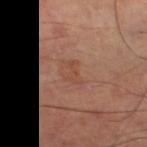| field | value |
|---|---|
| notes | no biopsy performed (imaged during a skin exam) |
| location | the left thigh |
| imaging modality | ~15 mm tile from a whole-body skin photo |
| patient | male, in their mid-60s |
| diameter | about 2.5 mm |
| tile lighting | cross-polarized illumination |